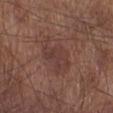Captured during whole-body skin photography for melanoma surveillance; the lesion was not biopsied. The subject is a male roughly 55 years of age. Located on the right lower leg. Imaged with white-light lighting. The lesion's longest dimension is about 5 mm. The lesion-visualizer software estimated a border-irregularity index near 3.5/10, internal color variation of about 2.5 on a 0–10 scale, and radial color variation of about 1. Cropped from a whole-body photographic skin survey; the tile spans about 15 mm.